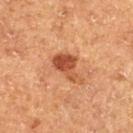Q: How large is the lesion?
A: ≈4.5 mm
Q: What kind of image is this?
A: ~15 mm tile from a whole-body skin photo
Q: Lesion location?
A: the left thigh
Q: What are the patient's age and sex?
A: male, about 75 years old
Q: How was the tile lit?
A: cross-polarized illumination